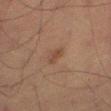Assessment: The lesion was photographed on a routine skin check and not biopsied; there is no pathology result. Acquisition and patient details: The subject is a male in their mid- to late 60s. The lesion-visualizer software estimated a lesion area of about 3 mm², an eccentricity of roughly 0.85, and a shape-asymmetry score of about 0.2 (0 = symmetric). The software also gave a border-irregularity rating of about 2/10, a within-lesion color-variation index near 1.5/10, and radial color variation of about 0.5. The analysis additionally found a classifier nevus-likeness of about 10/100 and lesion-presence confidence of about 100/100. On the left thigh. Captured under cross-polarized illumination. The lesion's longest dimension is about 2.5 mm. A region of skin cropped from a whole-body photographic capture, roughly 15 mm wide.